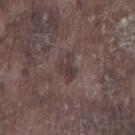<tbp_lesion>
  <biopsy_status>not biopsied; imaged during a skin examination</biopsy_status>
  <lesion_size>
    <long_diameter_mm_approx>3.5</long_diameter_mm_approx>
  </lesion_size>
  <site>right lower leg</site>
  <patient>
    <sex>male</sex>
    <age_approx>75</age_approx>
  </patient>
  <image>
    <source>total-body photography crop</source>
    <field_of_view_mm>15</field_of_view_mm>
  </image>
  <lighting>white-light</lighting>
</tbp_lesion>